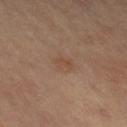A close-up tile cropped from a whole-body skin photograph, about 15 mm across. On the right leg. Automated image analysis of the tile measured an area of roughly 3.5 mm², an outline eccentricity of about 0.7 (0 = round, 1 = elongated), and a symmetry-axis asymmetry near 0.2. The software also gave a mean CIELAB color near L≈48 a*≈17 b*≈29, about 5 CIELAB-L* units darker than the surrounding skin, and a normalized lesion–skin contrast near 4.5. A female patient, about 60 years old. The recorded lesion diameter is about 2.5 mm.